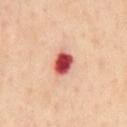This lesion was catalogued during total-body skin photography and was not selected for biopsy. A roughly 15 mm field-of-view crop from a total-body skin photograph. On the mid back. The total-body-photography lesion software estimated an area of roughly 6 mm², an eccentricity of roughly 0.75, and two-axis asymmetry of about 0.2. The analysis additionally found a lesion color around L≈53 a*≈38 b*≈30 in CIELAB and about 24 CIELAB-L* units darker than the surrounding skin. The software also gave a border-irregularity rating of about 1.5/10 and a peripheral color-asymmetry measure near 1.5. The software also gave a detector confidence of about 100 out of 100 that the crop contains a lesion. The lesion's longest dimension is about 3.5 mm. The subject is a male aged around 55.